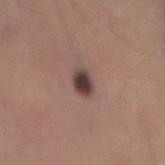Clinical impression:
The lesion was photographed on a routine skin check and not biopsied; there is no pathology result.
Background:
A male patient aged around 40. The total-body-photography lesion software estimated a lesion area of about 5 mm², an outline eccentricity of about 0.65 (0 = round, 1 = elongated), and two-axis asymmetry of about 0.2. The software also gave border irregularity of about 1.5 on a 0–10 scale and a within-lesion color-variation index near 4/10. A lesion tile, about 15 mm wide, cut from a 3D total-body photograph. The tile uses white-light illumination. From the mid back. Longest diameter approximately 3 mm.